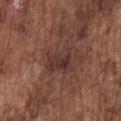| field | value |
|---|---|
| biopsy status | catalogued during a skin exam; not biopsied |
| image source | 15 mm crop, total-body photography |
| subject | male, about 75 years old |
| illumination | white-light |
| site | the chest |
| diameter | ≈4 mm |